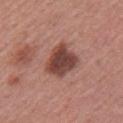Impression:
The lesion was photographed on a routine skin check and not biopsied; there is no pathology result.
Background:
A 15 mm crop from a total-body photograph taken for skin-cancer surveillance. The patient is a female roughly 50 years of age. Approximately 4 mm at its widest. The tile uses white-light illumination. Located on the left upper arm.